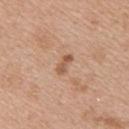Findings:
* follow-up · imaged on a skin check; not biopsied
* patient · female, about 45 years old
* image source · 15 mm crop, total-body photography
* lesion size · about 2.5 mm
* location · the upper back
* illumination · white-light illumination